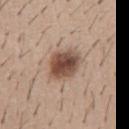| field | value |
|---|---|
| follow-up | total-body-photography surveillance lesion; no biopsy |
| location | the abdomen |
| subject | male, in their 40s |
| imaging modality | total-body-photography crop, ~15 mm field of view |